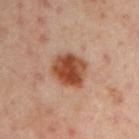* notes: no biopsy performed (imaged during a skin exam)
* patient: male, aged around 50
* image source: ~15 mm crop, total-body skin-cancer survey
* tile lighting: cross-polarized illumination
* location: the left upper arm